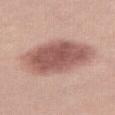Q: Patient demographics?
A: female, about 20 years old
Q: How was this image acquired?
A: 15 mm crop, total-body photography
Q: Where on the body is the lesion?
A: the right thigh
Q: What did automated image analysis measure?
A: an area of roughly 30 mm² and a shape eccentricity near 0.85; an average lesion color of about L≈55 a*≈23 b*≈24 (CIELAB), a lesion–skin lightness drop of about 15, and a lesion-to-skin contrast of about 9.5 (normalized; higher = more distinct)
Q: Illumination type?
A: white-light